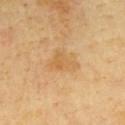Assessment:
No biopsy was performed on this lesion — it was imaged during a full skin examination and was not determined to be concerning.
Clinical summary:
On the chest. The tile uses cross-polarized illumination. Longest diameter approximately 3.5 mm. A region of skin cropped from a whole-body photographic capture, roughly 15 mm wide. The subject is a male aged approximately 65.